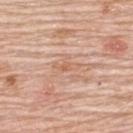Assessment:
Recorded during total-body skin imaging; not selected for excision or biopsy.
Acquisition and patient details:
A lesion tile, about 15 mm wide, cut from a 3D total-body photograph. A female patient roughly 65 years of age. Measured at roughly 3.5 mm in maximum diameter. Automated image analysis of the tile measured a lesion area of about 3 mm² and a symmetry-axis asymmetry near 0.45. Located on the upper back. Imaged with white-light lighting.